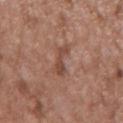Assessment: Recorded during total-body skin imaging; not selected for excision or biopsy. Image and clinical context: Captured under white-light illumination. Longest diameter approximately 3.5 mm. A male patient, roughly 50 years of age. This image is a 15 mm lesion crop taken from a total-body photograph. An algorithmic analysis of the crop reported a mean CIELAB color near L≈46 a*≈21 b*≈27 and roughly 9 lightness units darker than nearby skin. The analysis additionally found a border-irregularity index near 6/10 and a color-variation rating of about 0/10. On the mid back.